The total-body-photography lesion software estimated a shape eccentricity near 0.65 and a symmetry-axis asymmetry near 0.6. The analysis additionally found an average lesion color of about L≈49 a*≈26 b*≈26 (CIELAB) and roughly 8 lightness units darker than nearby skin. It also reported an automated nevus-likeness rating near 0 out of 100 and a detector confidence of about 95 out of 100 that the crop contains a lesion, the lesion is located on the head or neck, the recorded lesion diameter is about 4 mm, the patient is a male approximately 75 years of age, cropped from a whole-body photographic skin survey; the tile spans about 15 mm:
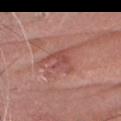Histopathologically confirmed as a nodular basal cell carcinoma (malignant).